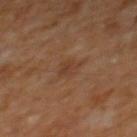  biopsy_status: not biopsied; imaged during a skin examination